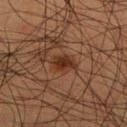follow-up: imaged on a skin check; not biopsied
size: about 3.5 mm
anatomic site: the right lower leg
patient: male, aged 48 to 52
imaging modality: ~15 mm tile from a whole-body skin photo
lighting: cross-polarized